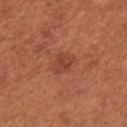Imaged during a routine full-body skin examination; the lesion was not biopsied and no histopathology is available. A female subject roughly 65 years of age. Imaged with white-light lighting. A 15 mm close-up tile from a total-body photography series done for melanoma screening. From the right upper arm. Longest diameter approximately 2.5 mm.